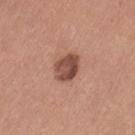<lesion>
<site>left thigh</site>
<lesion_size>
  <long_diameter_mm_approx>3.5</long_diameter_mm_approx>
</lesion_size>
<lighting>white-light</lighting>
<patient>
  <sex>female</sex>
  <age_approx>35</age_approx>
</patient>
<image>
  <source>total-body photography crop</source>
  <field_of_view_mm>15</field_of_view_mm>
</image>
</lesion>